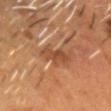Captured during whole-body skin photography for melanoma surveillance; the lesion was not biopsied.
From the head or neck.
The patient is a male aged around 55.
A 15 mm close-up tile from a total-body photography series done for melanoma screening.